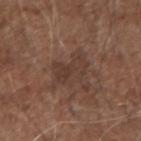Acquisition and patient details:
Automated tile analysis of the lesion measured an outline eccentricity of about 0.85 (0 = round, 1 = elongated) and two-axis asymmetry of about 0.45. It also reported an average lesion color of about L≈38 a*≈18 b*≈23 (CIELAB), roughly 6 lightness units darker than nearby skin, and a normalized lesion–skin contrast near 6. It also reported border irregularity of about 5.5 on a 0–10 scale, a color-variation rating of about 3/10, and peripheral color asymmetry of about 1. And it measured a nevus-likeness score of about 0/100. The recorded lesion diameter is about 5.5 mm. A 15 mm close-up extracted from a 3D total-body photography capture. From the left forearm. Captured under white-light illumination. A male patient, aged around 75.